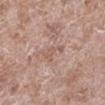Impression:
This lesion was catalogued during total-body skin photography and was not selected for biopsy.
Acquisition and patient details:
A lesion tile, about 15 mm wide, cut from a 3D total-body photograph. The tile uses white-light illumination. A male subject in their 60s. Automated tile analysis of the lesion measured a mean CIELAB color near L≈57 a*≈19 b*≈25, a lesion–skin lightness drop of about 7, and a lesion-to-skin contrast of about 4.5 (normalized; higher = more distinct). And it measured border irregularity of about 7 on a 0–10 scale and a peripheral color-asymmetry measure near 0. On the left lower leg.